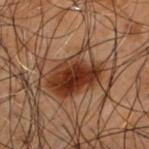Case summary:
- follow-up: imaged on a skin check; not biopsied
- illumination: cross-polarized illumination
- imaging modality: 15 mm crop, total-body photography
- subject: male, aged around 50
- lesion diameter: ≈5.5 mm
- site: the mid back